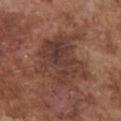| field | value |
|---|---|
| workup | total-body-photography surveillance lesion; no biopsy |
| image | total-body-photography crop, ~15 mm field of view |
| patient | male, roughly 75 years of age |
| lighting | white-light |
| site | the front of the torso |